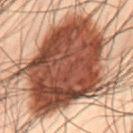Notes:
* follow-up: no biopsy performed (imaged during a skin exam)
* size: about 10.5 mm
* image: total-body-photography crop, ~15 mm field of view
* automated lesion analysis: a footprint of about 80 mm² and an eccentricity of roughly 0.5; about 18 CIELAB-L* units darker than the surrounding skin and a normalized lesion–skin contrast near 14.5; internal color variation of about 6 on a 0–10 scale and radial color variation of about 2
* tile lighting: cross-polarized illumination
* patient: male, approximately 50 years of age
* anatomic site: the lower back